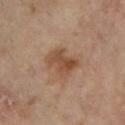{
  "image": {
    "source": "total-body photography crop",
    "field_of_view_mm": 15
  },
  "patient": {
    "sex": "female",
    "age_approx": 75
  },
  "lighting": "cross-polarized",
  "site": "right lower leg",
  "automated_metrics": {
    "area_mm2_approx": 9.0,
    "eccentricity": 0.65,
    "shape_asymmetry": 0.25,
    "border_irregularity_0_10": 3.0,
    "color_variation_0_10": 4.5,
    "peripheral_color_asymmetry": 1.5
  },
  "lesion_size": {
    "long_diameter_mm_approx": 3.5
  }
}